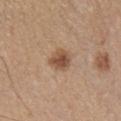Impression: The lesion was tiled from a total-body skin photograph and was not biopsied. Acquisition and patient details: A roughly 15 mm field-of-view crop from a total-body skin photograph. The lesion is on the right lower leg. A male subject aged approximately 45.